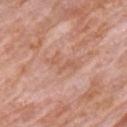biopsy_status: not biopsied; imaged during a skin examination
image:
  source: total-body photography crop
  field_of_view_mm: 15
patient:
  sex: male
  age_approx: 80
lesion_size:
  long_diameter_mm_approx: 4.0
site: chest